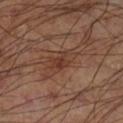The lesion was tiled from a total-body skin photograph and was not biopsied. A roughly 15 mm field-of-view crop from a total-body skin photograph. On the right thigh. Measured at roughly 3.5 mm in maximum diameter. This is a cross-polarized tile.